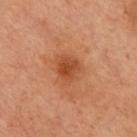| feature | finding |
|---|---|
| notes | imaged on a skin check; not biopsied |
| lesion size | ≈2.5 mm |
| lighting | cross-polarized |
| image | ~15 mm tile from a whole-body skin photo |
| body site | the chest |
| subject | female, in their mid- to late 50s |